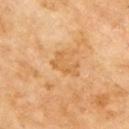<tbp_lesion>
<patient>
  <sex>male</sex>
  <age_approx>70</age_approx>
</patient>
<lighting>cross-polarized</lighting>
<image>
  <source>total-body photography crop</source>
  <field_of_view_mm>15</field_of_view_mm>
</image>
<site>right upper arm</site>
<lesion_size>
  <long_diameter_mm_approx>3.5</long_diameter_mm_approx>
</lesion_size>
</tbp_lesion>